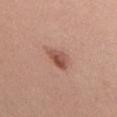Part of a total-body skin-imaging series; this lesion was reviewed on a skin check and was not flagged for biopsy. This image is a 15 mm lesion crop taken from a total-body photograph. Measured at roughly 3.5 mm in maximum diameter. The lesion-visualizer software estimated a normalized border contrast of about 8. The patient is a male in their mid-50s. Imaged with white-light lighting. The lesion is located on the upper back.